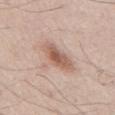The lesion was photographed on a routine skin check and not biopsied; there is no pathology result.
A region of skin cropped from a whole-body photographic capture, roughly 15 mm wide.
Located on the chest.
The tile uses white-light illumination.
A male patient, roughly 55 years of age.